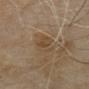Findings:
* follow-up — imaged on a skin check; not biopsied
* body site — the mid back
* diameter — ≈4 mm
* tile lighting — cross-polarized
* subject — male, aged around 60
* image source — ~15 mm crop, total-body skin-cancer survey
* image-analysis metrics — a lesion area of about 6 mm², a shape eccentricity near 0.9, and a shape-asymmetry score of about 0.3 (0 = symmetric); a lesion color around L≈42 a*≈13 b*≈29 in CIELAB and about 6 CIELAB-L* units darker than the surrounding skin; border irregularity of about 3.5 on a 0–10 scale and a peripheral color-asymmetry measure near 1; a classifier nevus-likeness of about 0/100 and a detector confidence of about 65 out of 100 that the crop contains a lesion